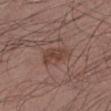Part of a total-body skin-imaging series; this lesion was reviewed on a skin check and was not flagged for biopsy. The tile uses white-light illumination. The subject is a male aged around 40. The lesion is located on the leg. This image is a 15 mm lesion crop taken from a total-body photograph.